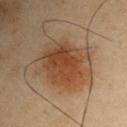Findings:
– subject · male, in their mid-50s
– image · ~15 mm tile from a whole-body skin photo
– lesion diameter · ~7 mm (longest diameter)
– tile lighting · cross-polarized illumination
– image-analysis metrics · a footprint of about 28 mm² and a symmetry-axis asymmetry near 0.2; a mean CIELAB color near L≈36 a*≈16 b*≈27, about 8 CIELAB-L* units darker than the surrounding skin, and a normalized border contrast of about 8; a border-irregularity rating of about 3/10, a color-variation rating of about 5/10, and peripheral color asymmetry of about 1.5; an automated nevus-likeness rating near 100 out of 100 and lesion-presence confidence of about 100/100
– anatomic site · the left upper arm